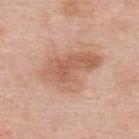Q: Is there a histopathology result?
A: imaged on a skin check; not biopsied
Q: Patient demographics?
A: female, aged 48–52
Q: What is the imaging modality?
A: 15 mm crop, total-body photography
Q: How large is the lesion?
A: about 7.5 mm
Q: Illumination type?
A: white-light illumination
Q: Automated lesion metrics?
A: an area of roughly 21 mm², an outline eccentricity of about 0.8 (0 = round, 1 = elongated), and a symmetry-axis asymmetry near 0.3; an average lesion color of about L≈60 a*≈22 b*≈31 (CIELAB) and a lesion–skin lightness drop of about 10
Q: Where on the body is the lesion?
A: the back